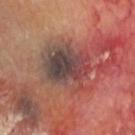Clinical impression:
Captured during whole-body skin photography for melanoma surveillance; the lesion was not biopsied.
Image and clinical context:
Imaged with cross-polarized lighting. Longest diameter approximately 6.5 mm. A close-up tile cropped from a whole-body skin photograph, about 15 mm across. A male patient, roughly 65 years of age. The lesion is located on the head or neck.